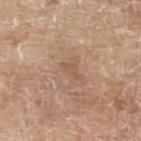The lesion was photographed on a routine skin check and not biopsied; there is no pathology result.
Measured at roughly 2.5 mm in maximum diameter.
The lesion is located on the left upper arm.
The lesion-visualizer software estimated an area of roughly 2.5 mm², an outline eccentricity of about 0.85 (0 = round, 1 = elongated), and a symmetry-axis asymmetry near 0.5. The analysis additionally found a lesion color around L≈55 a*≈19 b*≈30 in CIELAB, roughly 6 lightness units darker than nearby skin, and a normalized lesion–skin contrast near 4.5. And it measured a classifier nevus-likeness of about 0/100 and lesion-presence confidence of about 100/100.
The patient is a male about 80 years old.
A 15 mm close-up extracted from a 3D total-body photography capture.